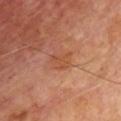biopsy status: catalogued during a skin exam; not biopsied | patient: male, aged around 70 | TBP lesion metrics: an area of roughly 4.5 mm², an eccentricity of roughly 0.6, and a symmetry-axis asymmetry near 0.3; internal color variation of about 2.5 on a 0–10 scale and peripheral color asymmetry of about 1; a nevus-likeness score of about 0/100 and a lesion-detection confidence of about 100/100 | location: the chest | image: 15 mm crop, total-body photography | lesion size: ≈3 mm.